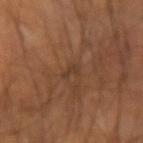Q: Was this lesion biopsied?
A: imaged on a skin check; not biopsied
Q: How was the tile lit?
A: cross-polarized illumination
Q: How large is the lesion?
A: about 2.5 mm
Q: What are the patient's age and sex?
A: male, aged 63–67
Q: What did automated image analysis measure?
A: a lesion area of about 2 mm², an eccentricity of roughly 0.9, and a shape-asymmetry score of about 0.5 (0 = symmetric); a lesion-to-skin contrast of about 5.5 (normalized; higher = more distinct)
Q: How was this image acquired?
A: ~15 mm tile from a whole-body skin photo
Q: Where on the body is the lesion?
A: the arm